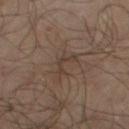Assessment: The lesion was tiled from a total-body skin photograph and was not biopsied. Background: A male subject, aged 63 to 67. Measured at roughly 3.5 mm in maximum diameter. Cropped from a whole-body photographic skin survey; the tile spans about 15 mm. Located on the left thigh. This is a cross-polarized tile. An algorithmic analysis of the crop reported a footprint of about 3.5 mm², a shape eccentricity near 0.9, and a shape-asymmetry score of about 0.7 (0 = symmetric). The analysis additionally found a lesion color around L≈35 a*≈12 b*≈21 in CIELAB and a lesion–skin lightness drop of about 5. The software also gave a border-irregularity index near 9/10, a within-lesion color-variation index near 0/10, and peripheral color asymmetry of about 0. The analysis additionally found a nevus-likeness score of about 0/100 and a lesion-detection confidence of about 60/100.